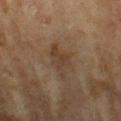Q: Was a biopsy performed?
A: imaged on a skin check; not biopsied
Q: What is the anatomic site?
A: the arm
Q: What kind of image is this?
A: 15 mm crop, total-body photography
Q: Who is the patient?
A: female, about 80 years old
Q: Automated lesion metrics?
A: a lesion area of about 6 mm² and an outline eccentricity of about 0.9 (0 = round, 1 = elongated); a lesion color around L≈33 a*≈13 b*≈24 in CIELAB, a lesion–skin lightness drop of about 6, and a normalized lesion–skin contrast near 6; an automated nevus-likeness rating near 0 out of 100 and lesion-presence confidence of about 95/100
Q: What is the lesion's diameter?
A: ≈5 mm